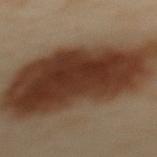Recorded during total-body skin imaging; not selected for excision or biopsy. The lesion is located on the upper back. The subject is a female aged 58 to 62. Imaged with cross-polarized lighting. Longest diameter approximately 14 mm. A region of skin cropped from a whole-body photographic capture, roughly 15 mm wide. Automated image analysis of the tile measured an area of roughly 80 mm², an eccentricity of roughly 0.85, and two-axis asymmetry of about 0.2. The software also gave an average lesion color of about L≈30 a*≈16 b*≈24 (CIELAB) and a normalized lesion–skin contrast near 13. It also reported a border-irregularity rating of about 3/10, internal color variation of about 5.5 on a 0–10 scale, and radial color variation of about 1.5.